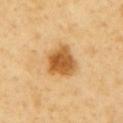Assessment:
No biopsy was performed on this lesion — it was imaged during a full skin examination and was not determined to be concerning.
Context:
A close-up tile cropped from a whole-body skin photograph, about 15 mm across. The patient is a female aged 68 to 72. The lesion is on the left upper arm.